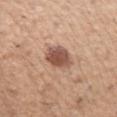Assessment: Part of a total-body skin-imaging series; this lesion was reviewed on a skin check and was not flagged for biopsy. Clinical summary: Measured at roughly 4 mm in maximum diameter. Cropped from a total-body skin-imaging series; the visible field is about 15 mm. The lesion is on the left upper arm. An algorithmic analysis of the crop reported a lesion-detection confidence of about 100/100. The subject is a male about 50 years old.